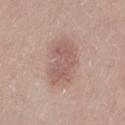Q: Was a biopsy performed?
A: catalogued during a skin exam; not biopsied
Q: How was the tile lit?
A: white-light
Q: How was this image acquired?
A: 15 mm crop, total-body photography
Q: Who is the patient?
A: female, approximately 50 years of age
Q: Lesion size?
A: ≈5 mm
Q: Where on the body is the lesion?
A: the left thigh